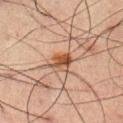Impression: Recorded during total-body skin imaging; not selected for excision or biopsy. Clinical summary: A close-up tile cropped from a whole-body skin photograph, about 15 mm across. Imaged with cross-polarized lighting. The total-body-photography lesion software estimated a lesion–skin lightness drop of about 10 and a lesion-to-skin contrast of about 9.5 (normalized; higher = more distinct). A male patient about 60 years old. Located on the abdomen. About 3 mm across.